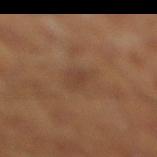Q: Was this lesion biopsied?
A: no biopsy performed (imaged during a skin exam)
Q: How large is the lesion?
A: about 3.5 mm
Q: Lesion location?
A: the leg
Q: What are the patient's age and sex?
A: male, aged 43 to 47
Q: How was the tile lit?
A: cross-polarized illumination
Q: How was this image acquired?
A: ~15 mm tile from a whole-body skin photo